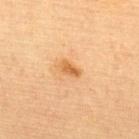<lesion>
<patient>
  <sex>female</sex>
  <age_approx>65</age_approx>
</patient>
<site>upper back</site>
<image>
  <source>total-body photography crop</source>
  <field_of_view_mm>15</field_of_view_mm>
</image>
</lesion>